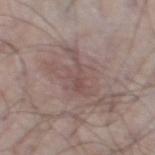<tbp_lesion>
  <biopsy_status>not biopsied; imaged during a skin examination</biopsy_status>
  <patient>
    <sex>male</sex>
    <age_approx>65</age_approx>
  </patient>
  <site>left thigh</site>
  <image>
    <source>total-body photography crop</source>
    <field_of_view_mm>15</field_of_view_mm>
  </image>
</tbp_lesion>